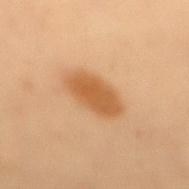Findings:
* biopsy status — catalogued during a skin exam; not biopsied
* acquisition — 15 mm crop, total-body photography
* body site — the mid back
* patient — female, aged around 40